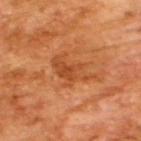No biopsy was performed on this lesion — it was imaged during a full skin examination and was not determined to be concerning. A male subject aged 63 to 67. Cropped from a whole-body photographic skin survey; the tile spans about 15 mm. This is a cross-polarized tile. The lesion's longest dimension is about 5 mm.